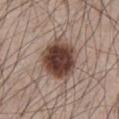Q: Was this lesion biopsied?
A: no biopsy performed (imaged during a skin exam)
Q: Patient demographics?
A: male, in their mid-60s
Q: Where on the body is the lesion?
A: the chest
Q: What kind of image is this?
A: ~15 mm crop, total-body skin-cancer survey